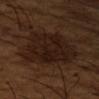Background: A 15 mm crop from a total-body photograph taken for skin-cancer surveillance. A male patient aged 63 to 67. Longest diameter approximately 7 mm. An algorithmic analysis of the crop reported a footprint of about 33 mm² and two-axis asymmetry of about 0.25. And it measured a lesion color around L≈18 a*≈15 b*≈20 in CIELAB and about 6 CIELAB-L* units darker than the surrounding skin. On the right upper arm. The tile uses cross-polarized illumination.